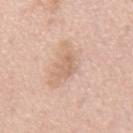workup = catalogued during a skin exam; not biopsied | subject = male, aged 63–67 | TBP lesion metrics = a nevus-likeness score of about 0/100 and a detector confidence of about 100 out of 100 that the crop contains a lesion | site = the mid back | image source = total-body-photography crop, ~15 mm field of view.